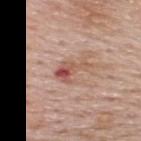The lesion was tiled from a total-body skin photograph and was not biopsied. Cropped from a total-body skin-imaging series; the visible field is about 15 mm. A male subject, about 60 years old. The recorded lesion diameter is about 4 mm. On the upper back.